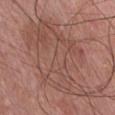biopsy_status: not biopsied; imaged during a skin examination
lighting: white-light
patient:
  sex: male
  age_approx: 70
image:
  source: total-body photography crop
  field_of_view_mm: 15
site: chest